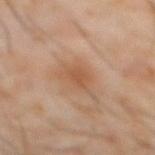Recorded during total-body skin imaging; not selected for excision or biopsy.
Imaged with cross-polarized lighting.
Cropped from a whole-body photographic skin survey; the tile spans about 15 mm.
The lesion is located on the abdomen.
A male subject, aged 58–62.
Automated tile analysis of the lesion measured a footprint of about 5 mm², an outline eccentricity of about 0.45 (0 = round, 1 = elongated), and a shape-asymmetry score of about 0.25 (0 = symmetric). The analysis additionally found a lesion color around L≈50 a*≈20 b*≈31 in CIELAB, a lesion–skin lightness drop of about 7, and a normalized lesion–skin contrast near 6.